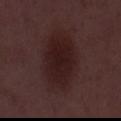– notes · total-body-photography surveillance lesion; no biopsy
– diameter · ≈8 mm
– automated metrics · an area of roughly 28 mm² and a shape-asymmetry score of about 0.1 (0 = symmetric); an average lesion color of about L≈20 a*≈18 b*≈15 (CIELAB), roughly 7 lightness units darker than nearby skin, and a normalized lesion–skin contrast near 8.5; border irregularity of about 1.5 on a 0–10 scale, a color-variation rating of about 2.5/10, and peripheral color asymmetry of about 1; a nevus-likeness score of about 65/100 and lesion-presence confidence of about 100/100
– subject · male, in their 50s
– illumination · white-light
– image source · ~15 mm tile from a whole-body skin photo